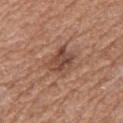- workup — total-body-photography surveillance lesion; no biopsy
- anatomic site — the right forearm
- subject — female, aged 68–72
- image source — 15 mm crop, total-body photography